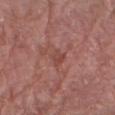- workup: no biopsy performed (imaged during a skin exam)
- subject: male, about 70 years old
- anatomic site: the right forearm
- image: ~15 mm tile from a whole-body skin photo
- tile lighting: white-light